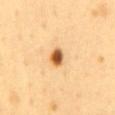{"biopsy_status": "not biopsied; imaged during a skin examination", "image": {"source": "total-body photography crop", "field_of_view_mm": 15}, "lighting": "cross-polarized", "patient": {"sex": "female", "age_approx": 50}, "lesion_size": {"long_diameter_mm_approx": 2.5}, "site": "abdomen"}